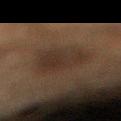Q: Was this lesion biopsied?
A: total-body-photography surveillance lesion; no biopsy
Q: Patient demographics?
A: male, aged 58 to 62
Q: What did automated image analysis measure?
A: a lesion color around L≈23 a*≈11 b*≈19 in CIELAB and a lesion–skin lightness drop of about 6; border irregularity of about 3 on a 0–10 scale and a peripheral color-asymmetry measure near 1
Q: What lighting was used for the tile?
A: cross-polarized
Q: What kind of image is this?
A: 15 mm crop, total-body photography
Q: What is the anatomic site?
A: the right lower leg
Q: How large is the lesion?
A: ~6.5 mm (longest diameter)